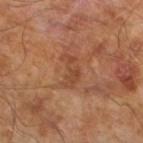notes: no biopsy performed (imaged during a skin exam) | patient: male, roughly 65 years of age | image source: ~15 mm tile from a whole-body skin photo | tile lighting: cross-polarized illumination | size: ~4 mm (longest diameter) | automated lesion analysis: a lesion area of about 6 mm², an outline eccentricity of about 0.85 (0 = round, 1 = elongated), and a symmetry-axis asymmetry near 0.45; border irregularity of about 5.5 on a 0–10 scale, a color-variation rating of about 3/10, and radial color variation of about 1.